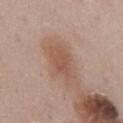Impression:
The lesion was tiled from a total-body skin photograph and was not biopsied.
Image and clinical context:
An algorithmic analysis of the crop reported a footprint of about 7.5 mm² and an eccentricity of roughly 0.85. It also reported a mean CIELAB color near L≈53 a*≈20 b*≈28, roughly 8 lightness units darker than nearby skin, and a normalized border contrast of about 6.5. The software also gave a border-irregularity rating of about 2/10, a color-variation rating of about 2.5/10, and radial color variation of about 1. The software also gave a classifier nevus-likeness of about 25/100 and lesion-presence confidence of about 100/100. About 4 mm across. A male patient approximately 55 years of age. A roughly 15 mm field-of-view crop from a total-body skin photograph. The lesion is on the mid back.